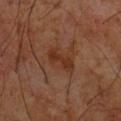| field | value |
|---|---|
| workup | total-body-photography surveillance lesion; no biopsy |
| body site | the back |
| diameter | about 3.5 mm |
| automated metrics | an area of roughly 5 mm², an eccentricity of roughly 0.9, and a symmetry-axis asymmetry near 0.3; an average lesion color of about L≈29 a*≈20 b*≈28 (CIELAB), a lesion–skin lightness drop of about 7, and a lesion-to-skin contrast of about 7.5 (normalized; higher = more distinct); a border-irregularity index near 4/10 and peripheral color asymmetry of about 1; a detector confidence of about 100 out of 100 that the crop contains a lesion |
| acquisition | ~15 mm tile from a whole-body skin photo |
| patient | male, aged 58–62 |
| lighting | cross-polarized |